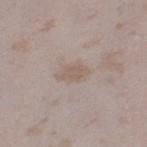Clinical impression: The lesion was photographed on a routine skin check and not biopsied; there is no pathology result. Acquisition and patient details: Measured at roughly 3.5 mm in maximum diameter. A female patient, approximately 25 years of age. An algorithmic analysis of the crop reported a lesion area of about 4 mm², an outline eccentricity of about 0.85 (0 = round, 1 = elongated), and a symmetry-axis asymmetry near 0.3. The software also gave a lesion color around L≈57 a*≈13 b*≈24 in CIELAB, a lesion–skin lightness drop of about 7, and a normalized border contrast of about 6. The analysis additionally found a border-irregularity index near 3.5/10. On the left thigh. This image is a 15 mm lesion crop taken from a total-body photograph. Captured under white-light illumination.